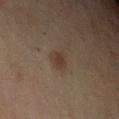Acquisition and patient details: On the arm. Imaged with cross-polarized lighting. The lesion-visualizer software estimated a lesion area of about 4.5 mm², an outline eccentricity of about 0.55 (0 = round, 1 = elongated), and a shape-asymmetry score of about 0.2 (0 = symmetric). The analysis additionally found a nevus-likeness score of about 70/100 and lesion-presence confidence of about 100/100. A female subject, aged 58–62. Measured at roughly 2.5 mm in maximum diameter. This image is a 15 mm lesion crop taken from a total-body photograph.